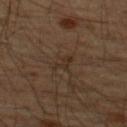• notes: catalogued during a skin exam; not biopsied
• size: ~3 mm (longest diameter)
• image-analysis metrics: a border-irregularity index near 5.5/10 and a color-variation rating of about 0/10
• patient: male, in their mid- to late 50s
• site: the upper back
• imaging modality: 15 mm crop, total-body photography